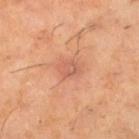Impression: Captured during whole-body skin photography for melanoma surveillance; the lesion was not biopsied. Acquisition and patient details: A male patient, aged 58–62. This image is a 15 mm lesion crop taken from a total-body photograph. From the left thigh.